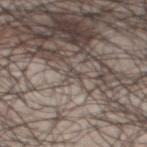Captured during whole-body skin photography for melanoma surveillance; the lesion was not biopsied. Located on the mid back. Cropped from a total-body skin-imaging series; the visible field is about 15 mm. The subject is a male in their 50s. About 1.5 mm across. Captured under white-light illumination.